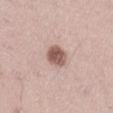No biopsy was performed on this lesion — it was imaged during a full skin examination and was not determined to be concerning. The lesion is located on the left thigh. Cropped from a total-body skin-imaging series; the visible field is about 15 mm. Longest diameter approximately 3 mm. A male subject, in their mid- to late 40s.